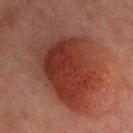  biopsy_status: not biopsied; imaged during a skin examination
  lesion_size:
    long_diameter_mm_approx: 10.5
  image:
    source: total-body photography crop
    field_of_view_mm: 15
  site: chest
  patient:
    sex: male
    age_approx: 40
  lighting: cross-polarized